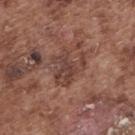The lesion was photographed on a routine skin check and not biopsied; there is no pathology result. An algorithmic analysis of the crop reported an area of roughly 10 mm², an eccentricity of roughly 0.6, and two-axis asymmetry of about 0.55. And it measured a mean CIELAB color near L≈41 a*≈20 b*≈24 and a normalized border contrast of about 7. It also reported a classifier nevus-likeness of about 0/100 and a lesion-detection confidence of about 70/100. The lesion is located on the upper back. Longest diameter approximately 5 mm. A 15 mm crop from a total-body photograph taken for skin-cancer surveillance. A male patient aged 73–77.